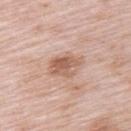Findings:
• follow-up — imaged on a skin check; not biopsied
• image — ~15 mm crop, total-body skin-cancer survey
• patient — female, aged approximately 65
• site — the upper back